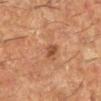follow-up = imaged on a skin check; not biopsied | lesion diameter = about 5.5 mm | site = the left lower leg | image source = total-body-photography crop, ~15 mm field of view | patient = female, aged 48 to 52 | lighting = cross-polarized.